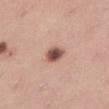anatomic site = the right thigh | patient = female, roughly 45 years of age | image-analysis metrics = a shape eccentricity near 0.6 and a symmetry-axis asymmetry near 0.15; an average lesion color of about L≈51 a*≈22 b*≈26 (CIELAB), a lesion–skin lightness drop of about 17, and a normalized border contrast of about 11.5; a detector confidence of about 100 out of 100 that the crop contains a lesion | illumination = white-light illumination | image = 15 mm crop, total-body photography | lesion diameter = ~2.5 mm (longest diameter).